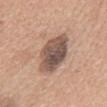Part of a total-body skin-imaging series; this lesion was reviewed on a skin check and was not flagged for biopsy. A female patient, roughly 55 years of age. This is a white-light tile. From the mid back. Approximately 5.5 mm at its widest. A region of skin cropped from a whole-body photographic capture, roughly 15 mm wide.